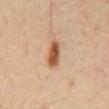Clinical impression:
Recorded during total-body skin imaging; not selected for excision or biopsy.
Acquisition and patient details:
A male subject aged 53 to 57. Measured at roughly 3.5 mm in maximum diameter. A close-up tile cropped from a whole-body skin photograph, about 15 mm across. Captured under cross-polarized illumination. The lesion is on the abdomen. Automated tile analysis of the lesion measured an outline eccentricity of about 0.85 (0 = round, 1 = elongated) and a symmetry-axis asymmetry near 0.15. And it measured an average lesion color of about L≈53 a*≈21 b*≈35 (CIELAB) and a lesion-to-skin contrast of about 10.5 (normalized; higher = more distinct). It also reported a lesion-detection confidence of about 100/100.